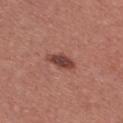Imaged during a routine full-body skin examination; the lesion was not biopsied and no histopathology is available. From the chest. A region of skin cropped from a whole-body photographic capture, roughly 15 mm wide. Longest diameter approximately 4 mm. A female subject, approximately 25 years of age.